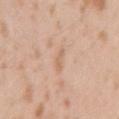Assessment:
This lesion was catalogued during total-body skin photography and was not selected for biopsy.
Background:
A roughly 15 mm field-of-view crop from a total-body skin photograph. The patient is a male aged 23 to 27. From the right upper arm.